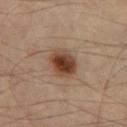Captured during whole-body skin photography for melanoma surveillance; the lesion was not biopsied. A male patient aged 53 to 57. From the left thigh. This image is a 15 mm lesion crop taken from a total-body photograph. Approximately 3.5 mm at its widest.